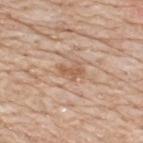Impression: Recorded during total-body skin imaging; not selected for excision or biopsy. Clinical summary: A 15 mm close-up tile from a total-body photography series done for melanoma screening. The tile uses white-light illumination. The lesion is located on the upper back. About 3 mm across. The lesion-visualizer software estimated a lesion area of about 4.5 mm², an outline eccentricity of about 0.75 (0 = round, 1 = elongated), and a shape-asymmetry score of about 0.35 (0 = symmetric). A male subject, aged around 80.